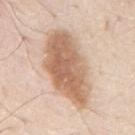The lesion is on the mid back. The patient is a male in their 80s. The recorded lesion diameter is about 9 mm. A close-up tile cropped from a whole-body skin photograph, about 15 mm across. Automated tile analysis of the lesion measured a lesion color around L≈64 a*≈18 b*≈31 in CIELAB and a normalized lesion–skin contrast near 9.